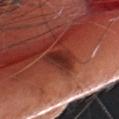biopsy status: no biopsy performed (imaged during a skin exam) | automated lesion analysis: an average lesion color of about L≈31 a*≈29 b*≈27 (CIELAB) and about 11 CIELAB-L* units darker than the surrounding skin; a border-irregularity rating of about 3/10, a color-variation rating of about 3.5/10, and a peripheral color-asymmetry measure near 1; an automated nevus-likeness rating near 0 out of 100 and a lesion-detection confidence of about 95/100 | size: about 4 mm | image source: total-body-photography crop, ~15 mm field of view | patient: female, aged approximately 55 | tile lighting: white-light | site: the head or neck.